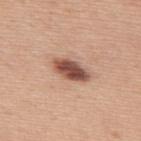Imaged during a routine full-body skin examination; the lesion was not biopsied and no histopathology is available.
Located on the upper back.
Approximately 4.5 mm at its widest.
A male patient aged approximately 70.
The tile uses white-light illumination.
Cropped from a whole-body photographic skin survey; the tile spans about 15 mm.
Automated tile analysis of the lesion measured a lesion area of about 7.5 mm² and an outline eccentricity of about 0.9 (0 = round, 1 = elongated). And it measured a lesion color around L≈50 a*≈23 b*≈27 in CIELAB, a lesion–skin lightness drop of about 18, and a normalized border contrast of about 12. It also reported a lesion-detection confidence of about 100/100.